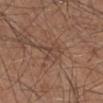notes: no biopsy performed (imaged during a skin exam)
lesion diameter: about 4 mm
image source: ~15 mm crop, total-body skin-cancer survey
patient: male, in their mid- to late 70s
illumination: white-light
automated lesion analysis: a footprint of about 7 mm², an outline eccentricity of about 0.75 (0 = round, 1 = elongated), and a shape-asymmetry score of about 0.35 (0 = symmetric); a mean CIELAB color near L≈44 a*≈17 b*≈26 and a lesion-to-skin contrast of about 4.5 (normalized; higher = more distinct); border irregularity of about 4 on a 0–10 scale, a color-variation rating of about 3/10, and a peripheral color-asymmetry measure near 1; an automated nevus-likeness rating near 0 out of 100 and a detector confidence of about 55 out of 100 that the crop contains a lesion
site: the right lower leg